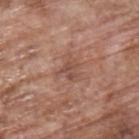* workup: no biopsy performed (imaged during a skin exam)
* site: the upper back
* subject: male, in their 70s
* image: 15 mm crop, total-body photography